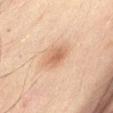| feature | finding |
|---|---|
| notes | imaged on a skin check; not biopsied |
| patient | female, roughly 60 years of age |
| body site | the front of the torso |
| lighting | cross-polarized illumination |
| acquisition | total-body-photography crop, ~15 mm field of view |